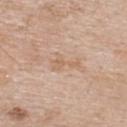* notes — no biopsy performed (imaged during a skin exam)
* patient — male, roughly 60 years of age
* image source — ~15 mm crop, total-body skin-cancer survey
* body site — the upper back